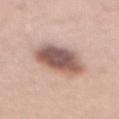Captured during whole-body skin photography for melanoma surveillance; the lesion was not biopsied. A 15 mm close-up tile from a total-body photography series done for melanoma screening. Automated image analysis of the tile measured a footprint of about 19 mm², an outline eccentricity of about 0.75 (0 = round, 1 = elongated), and two-axis asymmetry of about 0.15. The software also gave a lesion–skin lightness drop of about 17 and a normalized border contrast of about 10.5. A male patient aged approximately 40. About 6 mm across. From the abdomen. This is a white-light tile.